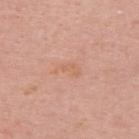Image and clinical context: Automated image analysis of the tile measured a lesion color around L≈63 a*≈23 b*≈33 in CIELAB, about 5 CIELAB-L* units darker than the surrounding skin, and a normalized border contrast of about 5. The analysis additionally found lesion-presence confidence of about 100/100. A 15 mm close-up tile from a total-body photography series done for melanoma screening. The tile uses white-light illumination. A male subject, roughly 65 years of age. The lesion is on the upper back. Approximately 3 mm at its widest.